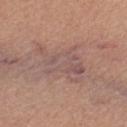No biopsy was performed on this lesion — it was imaged during a full skin examination and was not determined to be concerning. The patient is a female in their 60s. Located on the left thigh. This is a white-light tile. A 15 mm close-up extracted from a 3D total-body photography capture. About 7 mm across.